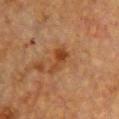Imaged during a routine full-body skin examination; the lesion was not biopsied and no histopathology is available. This is a cross-polarized tile. The recorded lesion diameter is about 4 mm. A female subject in their mid-50s. The lesion is on the front of the torso. Cropped from a whole-body photographic skin survey; the tile spans about 15 mm.